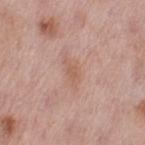Q: What are the patient's age and sex?
A: female, aged around 50
Q: Where on the body is the lesion?
A: the leg
Q: How large is the lesion?
A: about 4 mm
Q: What did automated image analysis measure?
A: a classifier nevus-likeness of about 0/100 and a lesion-detection confidence of about 100/100
Q: What kind of image is this?
A: ~15 mm crop, total-body skin-cancer survey